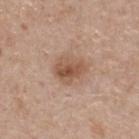The lesion was photographed on a routine skin check and not biopsied; there is no pathology result. A lesion tile, about 15 mm wide, cut from a 3D total-body photograph. Longest diameter approximately 3.5 mm. The subject is a male about 55 years old.